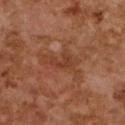| feature | finding |
|---|---|
| biopsy status | no biopsy performed (imaged during a skin exam) |
| lesion diameter | about 2.5 mm |
| patient | female, approximately 60 years of age |
| body site | the upper back |
| illumination | cross-polarized illumination |
| acquisition | ~15 mm crop, total-body skin-cancer survey |